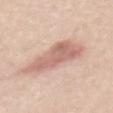Part of a total-body skin-imaging series; this lesion was reviewed on a skin check and was not flagged for biopsy.
A 15 mm close-up tile from a total-body photography series done for melanoma screening.
The patient is a male about 60 years old.
The lesion is located on the back.